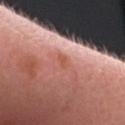biopsy status: no biopsy performed (imaged during a skin exam); location: the head or neck; tile lighting: white-light; lesion size: about 1.5 mm; subject: male, about 35 years old; imaging modality: ~15 mm tile from a whole-body skin photo.